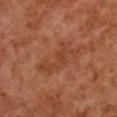Assessment: Recorded during total-body skin imaging; not selected for excision or biopsy. Clinical summary: Located on the chest. A female subject, in their 60s. A 15 mm close-up tile from a total-body photography series done for melanoma screening. The recorded lesion diameter is about 5.5 mm. Imaged with cross-polarized lighting.